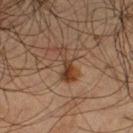Imaged during a routine full-body skin examination; the lesion was not biopsied and no histopathology is available.